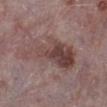Q: How was this image acquired?
A: ~15 mm crop, total-body skin-cancer survey
Q: Lesion location?
A: the left lower leg
Q: Patient demographics?
A: male, about 75 years old
Q: Lesion size?
A: ≈8.5 mm